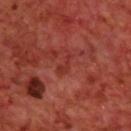Clinical impression:
Recorded during total-body skin imaging; not selected for excision or biopsy.
Clinical summary:
The tile uses cross-polarized illumination. A 15 mm close-up extracted from a 3D total-body photography capture. On the upper back. A male subject, aged around 70. The lesion's longest dimension is about 3 mm. The lesion-visualizer software estimated a lesion-to-skin contrast of about 5.5 (normalized; higher = more distinct). The analysis additionally found an automated nevus-likeness rating near 0 out of 100 and a detector confidence of about 85 out of 100 that the crop contains a lesion.